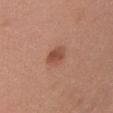Part of a total-body skin-imaging series; this lesion was reviewed on a skin check and was not flagged for biopsy.
The lesion is located on the chest.
A female patient about 35 years old.
The tile uses white-light illumination.
Automated tile analysis of the lesion measured an average lesion color of about L≈50 a*≈24 b*≈30 (CIELAB) and about 10 CIELAB-L* units darker than the surrounding skin.
A region of skin cropped from a whole-body photographic capture, roughly 15 mm wide.